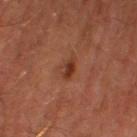| feature | finding |
|---|---|
| biopsy status | total-body-photography surveillance lesion; no biopsy |
| automated metrics | a mean CIELAB color near L≈33 a*≈23 b*≈28 and a normalized lesion–skin contrast near 7.5; a classifier nevus-likeness of about 75/100 and a lesion-detection confidence of about 100/100 |
| illumination | cross-polarized |
| image | 15 mm crop, total-body photography |
| patient | male, in their 60s |
| lesion size | ~2.5 mm (longest diameter) |
| location | the right thigh |